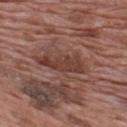No biopsy was performed on this lesion — it was imaged during a full skin examination and was not determined to be concerning.
Automated tile analysis of the lesion measured a border-irregularity rating of about 4.5/10, a color-variation rating of about 2.5/10, and a peripheral color-asymmetry measure near 0.5.
On the upper back.
About 6 mm across.
A 15 mm crop from a total-body photograph taken for skin-cancer surveillance.
A male subject, aged 68 to 72.